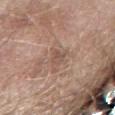workup = catalogued during a skin exam; not biopsied | lesion size = ≈2.5 mm | patient = male, aged 78 to 82 | location = the right forearm | tile lighting = white-light illumination | image source = ~15 mm crop, total-body skin-cancer survey.